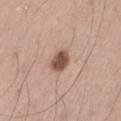<record>
<biopsy_status>not biopsied; imaged during a skin examination</biopsy_status>
<automated_metrics>
  <area_mm2_approx>6.0</area_mm2_approx>
  <eccentricity>0.55</eccentricity>
  <shape_asymmetry>0.2</shape_asymmetry>
  <lesion_detection_confidence_0_100>100</lesion_detection_confidence_0_100>
</automated_metrics>
<patient>
  <sex>male</sex>
  <age_approx>55</age_approx>
</patient>
<lighting>white-light</lighting>
<lesion_size>
  <long_diameter_mm_approx>3.0</long_diameter_mm_approx>
</lesion_size>
<site>left thigh</site>
<image>
  <source>total-body photography crop</source>
  <field_of_view_mm>15</field_of_view_mm>
</image>
</record>